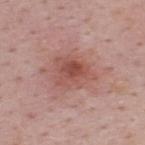notes: imaged on a skin check; not biopsied | image source: ~15 mm tile from a whole-body skin photo | anatomic site: the back | subject: male, aged 48 to 52.